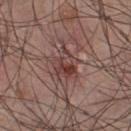Q: Is there a histopathology result?
A: no biopsy performed (imaged during a skin exam)
Q: Who is the patient?
A: male, in their mid- to late 20s
Q: How was this image acquired?
A: ~15 mm tile from a whole-body skin photo
Q: What is the anatomic site?
A: the chest